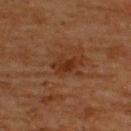Captured during whole-body skin photography for melanoma surveillance; the lesion was not biopsied.
Automated image analysis of the tile measured a lesion area of about 5 mm² and a symmetry-axis asymmetry near 0.3. It also reported a lesion–skin lightness drop of about 6 and a normalized border contrast of about 7. The analysis additionally found a within-lesion color-variation index near 3/10 and peripheral color asymmetry of about 1.
This is a cross-polarized tile.
Cropped from a whole-body photographic skin survey; the tile spans about 15 mm.
From the upper back.
A male patient, about 65 years old.
The recorded lesion diameter is about 3.5 mm.